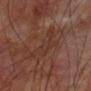Case summary:
• notes · no biopsy performed (imaged during a skin exam)
• lighting · cross-polarized illumination
• patient · male, approximately 70 years of age
• anatomic site · the left upper arm
• image source · 15 mm crop, total-body photography